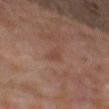Assessment:
This lesion was catalogued during total-body skin photography and was not selected for biopsy.